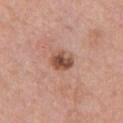Case summary:
• workup: no biopsy performed (imaged during a skin exam)
• imaging modality: ~15 mm tile from a whole-body skin photo
• anatomic site: the chest
• subject: male, in their 60s